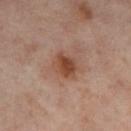No biopsy was performed on this lesion — it was imaged during a full skin examination and was not determined to be concerning. A roughly 15 mm field-of-view crop from a total-body skin photograph. The total-body-photography lesion software estimated a lesion area of about 7 mm² and an eccentricity of roughly 0.55. And it measured an automated nevus-likeness rating near 80 out of 100. A female patient about 55 years old. Imaged with cross-polarized lighting. The lesion is on the left thigh.